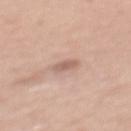Assessment:
The lesion was tiled from a total-body skin photograph and was not biopsied.
Clinical summary:
Longest diameter approximately 2.5 mm. A 15 mm close-up tile from a total-body photography series done for melanoma screening. The lesion-visualizer software estimated a normalized lesion–skin contrast near 6. It also reported a border-irregularity rating of about 2.5/10, a color-variation rating of about 1/10, and radial color variation of about 0. And it measured an automated nevus-likeness rating near 0 out of 100. The tile uses white-light illumination. A female patient aged around 45. On the mid back.